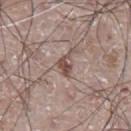notes = catalogued during a skin exam; not biopsied
lesion diameter = ≈2.5 mm
image = total-body-photography crop, ~15 mm field of view
site = the front of the torso
patient = male, in their mid- to late 70s